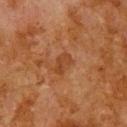Q: Was this lesion biopsied?
A: no biopsy performed (imaged during a skin exam)